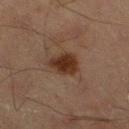The lesion was tiled from a total-body skin photograph and was not biopsied. The recorded lesion diameter is about 3.5 mm. A male patient, aged 63–67. Located on the left thigh. The total-body-photography lesion software estimated a lesion color around L≈25 a*≈16 b*≈23 in CIELAB. The software also gave a classifier nevus-likeness of about 95/100 and a detector confidence of about 100 out of 100 that the crop contains a lesion. A 15 mm close-up extracted from a 3D total-body photography capture. Imaged with cross-polarized lighting.